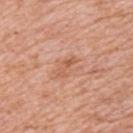Q: Was a biopsy performed?
A: catalogued during a skin exam; not biopsied
Q: What kind of image is this?
A: total-body-photography crop, ~15 mm field of view
Q: Where on the body is the lesion?
A: the upper back
Q: Lesion size?
A: about 3 mm
Q: Patient demographics?
A: female, aged 38–42
Q: What did automated image analysis measure?
A: a lesion area of about 3 mm² and an outline eccentricity of about 0.95 (0 = round, 1 = elongated); a mean CIELAB color near L≈58 a*≈25 b*≈33, about 8 CIELAB-L* units darker than the surrounding skin, and a normalized lesion–skin contrast near 5.5; a classifier nevus-likeness of about 0/100 and lesion-presence confidence of about 100/100